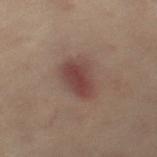A female subject approximately 60 years of age. Captured under cross-polarized illumination. A 15 mm crop from a total-body photograph taken for skin-cancer surveillance. The lesion is located on the right leg.